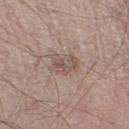Q: Was a biopsy performed?
A: catalogued during a skin exam; not biopsied
Q: Lesion size?
A: ≈3.5 mm
Q: Who is the patient?
A: male, in their 60s
Q: What is the imaging modality?
A: total-body-photography crop, ~15 mm field of view
Q: What did automated image analysis measure?
A: a nevus-likeness score of about 0/100 and a lesion-detection confidence of about 100/100
Q: Where on the body is the lesion?
A: the leg
Q: What lighting was used for the tile?
A: white-light